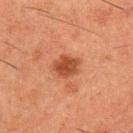follow-up: imaged on a skin check; not biopsied | lesion diameter: ~3 mm (longest diameter) | illumination: cross-polarized illumination | subject: male, about 50 years old | image-analysis metrics: a footprint of about 6.5 mm², a shape eccentricity near 0.55, and a symmetry-axis asymmetry near 0.2; a mean CIELAB color near L≈38 a*≈24 b*≈31, roughly 10 lightness units darker than nearby skin, and a normalized border contrast of about 8.5; border irregularity of about 2 on a 0–10 scale and peripheral color asymmetry of about 1 | acquisition: total-body-photography crop, ~15 mm field of view | location: the upper back.